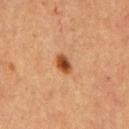Clinical summary:
The lesion's longest dimension is about 2.5 mm. The tile uses cross-polarized illumination. A 15 mm close-up extracted from a 3D total-body photography capture. From the chest. A male patient, aged 63 to 67.